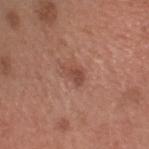Case summary:
- notes — imaged on a skin check; not biopsied
- tile lighting — white-light illumination
- imaging modality — total-body-photography crop, ~15 mm field of view
- site — the head or neck
- automated metrics — a lesion area of about 4 mm² and a shape-asymmetry score of about 0.4 (0 = symmetric); border irregularity of about 4 on a 0–10 scale, internal color variation of about 2 on a 0–10 scale, and radial color variation of about 0.5; a nevus-likeness score of about 60/100 and lesion-presence confidence of about 100/100
- subject — male, in their mid-30s